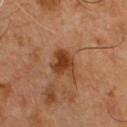Case summary:
* biopsy status — total-body-photography surveillance lesion; no biopsy
* lesion diameter — ~3 mm (longest diameter)
* lighting — cross-polarized illumination
* patient — male, in their 50s
* automated lesion analysis — a shape eccentricity near 0.45 and a symmetry-axis asymmetry near 0.3; a lesion color around L≈40 a*≈24 b*≈35 in CIELAB and a normalized border contrast of about 9.5; a border-irregularity rating of about 3.5/10, internal color variation of about 3.5 on a 0–10 scale, and radial color variation of about 1; an automated nevus-likeness rating near 95 out of 100
* imaging modality — ~15 mm tile from a whole-body skin photo
* body site — the front of the torso